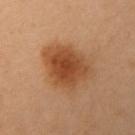{"biopsy_status": "not biopsied; imaged during a skin examination", "site": "right upper arm", "lesion_size": {"long_diameter_mm_approx": 6.0}, "patient": {"sex": "female", "age_approx": 30}, "automated_metrics": {"cielab_L": 45, "cielab_a": 22, "cielab_b": 35, "vs_skin_darker_L": 11.0, "peripheral_color_asymmetry": 1.5, "nevus_likeness_0_100": 100}, "lighting": "cross-polarized", "image": {"source": "total-body photography crop", "field_of_view_mm": 15}}